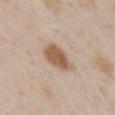Located on the front of the torso. A 15 mm crop from a total-body photograph taken for skin-cancer surveillance. The patient is a female aged 23 to 27.